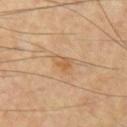Clinical impression: The lesion was tiled from a total-body skin photograph and was not biopsied. Image and clinical context: Longest diameter approximately 3 mm. A 15 mm close-up tile from a total-body photography series done for melanoma screening. On the chest. This is a cross-polarized tile. The patient is a male aged around 60.